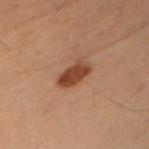Q: Was a biopsy performed?
A: imaged on a skin check; not biopsied